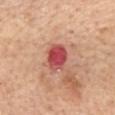The lesion was photographed on a routine skin check and not biopsied; there is no pathology result. A 15 mm crop from a total-body photograph taken for skin-cancer surveillance. A female patient, aged 63 to 67. Located on the mid back. Captured under white-light illumination. About 3.5 mm across.